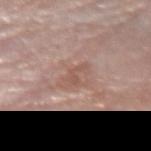<lesion>
  <biopsy_status>not biopsied; imaged during a skin examination</biopsy_status>
  <site>arm</site>
  <lesion_size>
    <long_diameter_mm_approx>3.0</long_diameter_mm_approx>
  </lesion_size>
  <lighting>white-light</lighting>
  <image>
    <source>total-body photography crop</source>
    <field_of_view_mm>15</field_of_view_mm>
  </image>
  <patient>
    <sex>female</sex>
    <age_approx>65</age_approx>
  </patient>
</lesion>